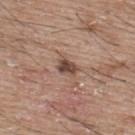No biopsy was performed on this lesion — it was imaged during a full skin examination and was not determined to be concerning. The lesion is on the upper back. A 15 mm crop from a total-body photograph taken for skin-cancer surveillance. A male patient, in their mid-60s. The recorded lesion diameter is about 3 mm.